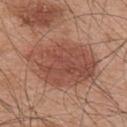{"biopsy_status": "not biopsied; imaged during a skin examination", "lesion_size": {"long_diameter_mm_approx": 8.0}, "site": "upper back", "lighting": "white-light", "patient": {"sex": "male", "age_approx": 45}, "image": {"source": "total-body photography crop", "field_of_view_mm": 15}, "automated_metrics": {"cielab_L": 49, "cielab_a": 24, "cielab_b": 28, "vs_skin_darker_L": 10.0, "vs_skin_contrast_norm": 7.5, "border_irregularity_0_10": 2.5, "color_variation_0_10": 4.5, "peripheral_color_asymmetry": 1.5, "nevus_likeness_0_100": 90}}